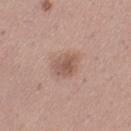This lesion was catalogued during total-body skin photography and was not selected for biopsy.
A region of skin cropped from a whole-body photographic capture, roughly 15 mm wide.
The patient is a female in their 30s.
This is a white-light tile.
Automated tile analysis of the lesion measured a lesion–skin lightness drop of about 10 and a normalized lesion–skin contrast near 6.5. And it measured border irregularity of about 3 on a 0–10 scale, a within-lesion color-variation index near 3.5/10, and peripheral color asymmetry of about 1.
Longest diameter approximately 3.5 mm.
Located on the left thigh.